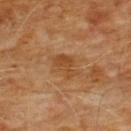Impression:
This lesion was catalogued during total-body skin photography and was not selected for biopsy.
Context:
About 3.5 mm across. From the chest. A region of skin cropped from a whole-body photographic capture, roughly 15 mm wide. The subject is a male about 60 years old.